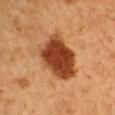Q: What lighting was used for the tile?
A: cross-polarized illumination
Q: Lesion location?
A: the left upper arm
Q: What did automated image analysis measure?
A: an area of roughly 22 mm², a shape eccentricity near 0.7, and a shape-asymmetry score of about 0.25 (0 = symmetric); a color-variation rating of about 5/10; lesion-presence confidence of about 100/100
Q: What is the imaging modality?
A: 15 mm crop, total-body photography
Q: Patient demographics?
A: male, roughly 50 years of age
Q: How large is the lesion?
A: about 6 mm